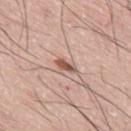Q: Is there a histopathology result?
A: catalogued during a skin exam; not biopsied